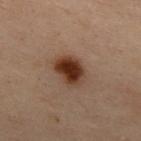| field | value |
|---|---|
| notes | no biopsy performed (imaged during a skin exam) |